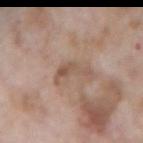Notes:
– notes: catalogued during a skin exam; not biopsied
– patient: male, approximately 60 years of age
– lighting: white-light illumination
– lesion diameter: ≈4.5 mm
– imaging modality: ~15 mm crop, total-body skin-cancer survey
– site: the left forearm